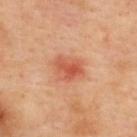Assessment:
Imaged during a routine full-body skin examination; the lesion was not biopsied and no histopathology is available.
Clinical summary:
The lesion is on the upper back. A close-up tile cropped from a whole-body skin photograph, about 15 mm across. A female subject aged around 40.